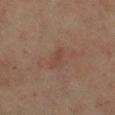Imaged with cross-polarized lighting.
The lesion's longest dimension is about 3 mm.
A close-up tile cropped from a whole-body skin photograph, about 15 mm across.
Automated tile analysis of the lesion measured internal color variation of about 0.5 on a 0–10 scale. The analysis additionally found an automated nevus-likeness rating near 0 out of 100 and lesion-presence confidence of about 100/100.
From the leg.
The patient is a female in their 70s.